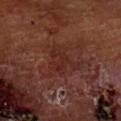<tbp_lesion>
  <biopsy_status>not biopsied; imaged during a skin examination</biopsy_status>
  <patient>
    <sex>male</sex>
    <age_approx>65</age_approx>
  </patient>
  <site>left arm</site>
  <image>
    <source>total-body photography crop</source>
    <field_of_view_mm>15</field_of_view_mm>
  </image>
  <lesion_size>
    <long_diameter_mm_approx>3.0</long_diameter_mm_approx>
  </lesion_size>
</tbp_lesion>